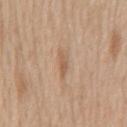{
  "lesion_size": {
    "long_diameter_mm_approx": 3.0
  },
  "patient": {
    "sex": "male",
    "age_approx": 60
  },
  "automated_metrics": {
    "area_mm2_approx": 2.5,
    "shape_asymmetry": 0.4,
    "cielab_L": 57,
    "cielab_a": 19,
    "cielab_b": 32,
    "vs_skin_darker_L": 9.0,
    "nevus_likeness_0_100": 0,
    "lesion_detection_confidence_0_100": 100
  },
  "image": {
    "source": "total-body photography crop",
    "field_of_view_mm": 15
  },
  "site": "back",
  "lighting": "white-light"
}